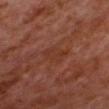The lesion's longest dimension is about 3 mm. A male subject, aged around 55. Cropped from a total-body skin-imaging series; the visible field is about 15 mm. Located on the head or neck. This is a cross-polarized tile.